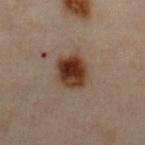Findings:
• notes · no biopsy performed (imaged during a skin exam)
• image source · total-body-photography crop, ~15 mm field of view
• diameter · ~4 mm (longest diameter)
• body site · the chest
• patient · male, aged approximately 50
• TBP lesion metrics · an outline eccentricity of about 0.45 (0 = round, 1 = elongated); an automated nevus-likeness rating near 100 out of 100 and a detector confidence of about 100 out of 100 that the crop contains a lesion